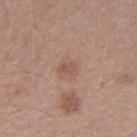{"biopsy_status": "not biopsied; imaged during a skin examination", "patient": {"sex": "male", "age_approx": 30}, "image": {"source": "total-body photography crop", "field_of_view_mm": 15}}